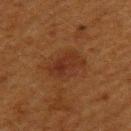{"biopsy_status": "not biopsied; imaged during a skin examination", "automated_metrics": {"area_mm2_approx": 11.0, "shape_asymmetry": 0.25, "cielab_L": 26, "cielab_a": 20, "cielab_b": 26, "vs_skin_contrast_norm": 6.0, "border_irregularity_0_10": 3.0, "color_variation_0_10": 3.5, "peripheral_color_asymmetry": 1.0}, "lesion_size": {"long_diameter_mm_approx": 4.5}, "image": {"source": "total-body photography crop", "field_of_view_mm": 15}, "patient": {"sex": "female", "age_approx": 40}, "site": "back"}